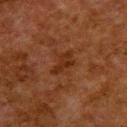Q: Lesion location?
A: the upper back
Q: How was this image acquired?
A: ~15 mm tile from a whole-body skin photo
Q: What did automated image analysis measure?
A: a lesion area of about 4.5 mm², an outline eccentricity of about 0.85 (0 = round, 1 = elongated), and a shape-asymmetry score of about 0.4 (0 = symmetric); an average lesion color of about L≈24 a*≈20 b*≈28 (CIELAB) and a lesion–skin lightness drop of about 6; a nevus-likeness score of about 0/100 and lesion-presence confidence of about 100/100
Q: Lesion size?
A: ≈3.5 mm
Q: Who is the patient?
A: female, roughly 50 years of age
Q: What lighting was used for the tile?
A: cross-polarized illumination